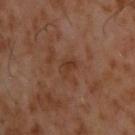Imaged during a routine full-body skin examination; the lesion was not biopsied and no histopathology is available. A lesion tile, about 15 mm wide, cut from a 3D total-body photograph. The lesion's longest dimension is about 2.5 mm. Located on the upper back. This is a cross-polarized tile. A male subject approximately 60 years of age.